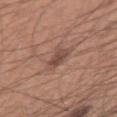This lesion was catalogued during total-body skin photography and was not selected for biopsy. Measured at roughly 3 mm in maximum diameter. A male patient, in their mid- to late 30s. Imaged with white-light lighting. A 15 mm close-up tile from a total-body photography series done for melanoma screening. The lesion is on the arm. An algorithmic analysis of the crop reported a mean CIELAB color near L≈47 a*≈19 b*≈24, a lesion–skin lightness drop of about 9, and a normalized border contrast of about 7. The analysis additionally found a border-irregularity index near 3/10, internal color variation of about 2.5 on a 0–10 scale, and a peripheral color-asymmetry measure near 1. The software also gave a classifier nevus-likeness of about 50/100 and a lesion-detection confidence of about 100/100.